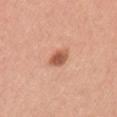Q: Was this lesion biopsied?
A: no biopsy performed (imaged during a skin exam)
Q: How was the tile lit?
A: white-light illumination
Q: What kind of image is this?
A: ~15 mm tile from a whole-body skin photo
Q: Lesion location?
A: the left upper arm
Q: Lesion size?
A: about 3 mm
Q: Who is the patient?
A: female, in their mid-20s